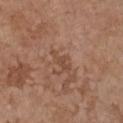This lesion was catalogued during total-body skin photography and was not selected for biopsy. A female subject, aged 63 to 67. About 3.5 mm across. Captured under white-light illumination. A roughly 15 mm field-of-view crop from a total-body skin photograph. Located on the chest. The total-body-photography lesion software estimated an area of roughly 3.5 mm², an outline eccentricity of about 0.9 (0 = round, 1 = elongated), and a symmetry-axis asymmetry near 0.4. And it measured an average lesion color of about L≈47 a*≈20 b*≈29 (CIELAB) and a lesion–skin lightness drop of about 7. The software also gave a border-irregularity index near 5/10 and a within-lesion color-variation index near 1/10.